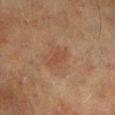No biopsy was performed on this lesion — it was imaged during a full skin examination and was not determined to be concerning. Approximately 3.5 mm at its widest. The total-body-photography lesion software estimated a footprint of about 3.5 mm², a shape eccentricity near 0.9, and two-axis asymmetry of about 0.25. The analysis additionally found a mean CIELAB color near L≈38 a*≈20 b*≈26, about 5 CIELAB-L* units darker than the surrounding skin, and a lesion-to-skin contrast of about 5 (normalized; higher = more distinct). It also reported a border-irregularity rating of about 3/10, internal color variation of about 1 on a 0–10 scale, and a peripheral color-asymmetry measure near 0.5. The analysis additionally found a nevus-likeness score of about 75/100 and a lesion-detection confidence of about 100/100. The lesion is on the right lower leg. A region of skin cropped from a whole-body photographic capture, roughly 15 mm wide. A male patient aged 68–72.